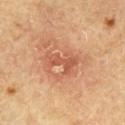{"biopsy_status": "not biopsied; imaged during a skin examination", "lighting": "cross-polarized", "patient": {"sex": "male", "age_approx": 65}, "site": "chest", "image": {"source": "total-body photography crop", "field_of_view_mm": 15}, "lesion_size": {"long_diameter_mm_approx": 4.0}}